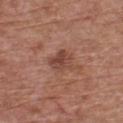Findings:
– workup: catalogued during a skin exam; not biopsied
– diameter: ~3 mm (longest diameter)
– image source: 15 mm crop, total-body photography
– anatomic site: the chest
– subject: male, about 75 years old